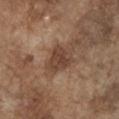The lesion was tiled from a total-body skin photograph and was not biopsied. A male subject aged around 75. A lesion tile, about 15 mm wide, cut from a 3D total-body photograph. Located on the chest.